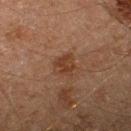Approximately 3 mm at its widest.
A close-up tile cropped from a whole-body skin photograph, about 15 mm across.
Captured under cross-polarized illumination.
The lesion is located on the right lower leg.
The patient is a male aged 58–62.